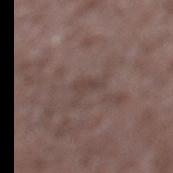<lesion>
<patient>
  <sex>male</sex>
  <age_approx>70</age_approx>
</patient>
<site>leg</site>
<image>
  <source>total-body photography crop</source>
  <field_of_view_mm>15</field_of_view_mm>
</image>
<automated_metrics>
  <cielab_L>40</cielab_L>
  <cielab_a>15</cielab_a>
  <cielab_b>19</cielab_b>
  <vs_skin_darker_L>6.0</vs_skin_darker_L>
  <vs_skin_contrast_norm>5.0</vs_skin_contrast_norm>
  <nevus_likeness_0_100>0</nevus_likeness_0_100>
</automated_metrics>
<lesion_size>
  <long_diameter_mm_approx>2.5</long_diameter_mm_approx>
</lesion_size>
</lesion>